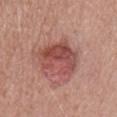Part of a total-body skin-imaging series; this lesion was reviewed on a skin check and was not flagged for biopsy. The patient is a male in their 50s. From the mid back. A close-up tile cropped from a whole-body skin photograph, about 15 mm across. Measured at roughly 5 mm in maximum diameter. Captured under white-light illumination.